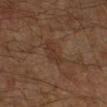– follow-up · imaged on a skin check; not biopsied
– lesion diameter · about 3 mm
– TBP lesion metrics · an area of roughly 4 mm² and two-axis asymmetry of about 0.4; a lesion color around L≈29 a*≈16 b*≈25 in CIELAB, a lesion–skin lightness drop of about 5, and a lesion-to-skin contrast of about 6 (normalized; higher = more distinct); a nevus-likeness score of about 0/100 and lesion-presence confidence of about 100/100
– subject · male, aged approximately 60
– image source · ~15 mm crop, total-body skin-cancer survey
– anatomic site · the right lower leg
– lighting · cross-polarized illumination